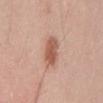Findings:
• notes · imaged on a skin check; not biopsied
• illumination · white-light
• anatomic site · the mid back
• subject · male, about 35 years old
• lesion diameter · ≈3.5 mm
• automated lesion analysis · an area of roughly 7.5 mm²; an average lesion color of about L≈57 a*≈23 b*≈29 (CIELAB) and a lesion–skin lightness drop of about 12; an automated nevus-likeness rating near 95 out of 100 and lesion-presence confidence of about 100/100
• acquisition · ~15 mm tile from a whole-body skin photo